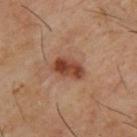Imaged during a routine full-body skin examination; the lesion was not biopsied and no histopathology is available. About 3.5 mm across. This image is a 15 mm lesion crop taken from a total-body photograph. This is a cross-polarized tile. A male subject approximately 65 years of age. From the upper back.